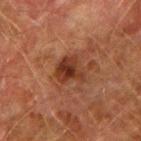<tbp_lesion>
<automated_metrics>
  <cielab_L>30</cielab_L>
  <cielab_a>21</cielab_a>
  <cielab_b>27</cielab_b>
  <vs_skin_darker_L>9.0</vs_skin_darker_L>
  <vs_skin_contrast_norm>9.0</vs_skin_contrast_norm>
  <nevus_likeness_0_100>80</nevus_likeness_0_100>
  <lesion_detection_confidence_0_100>100</lesion_detection_confidence_0_100>
</automated_metrics>
<site>right upper arm</site>
<image>
  <source>total-body photography crop</source>
  <field_of_view_mm>15</field_of_view_mm>
</image>
<patient>
  <sex>male</sex>
  <age_approx>75</age_approx>
</patient>
<lesion_size>
  <long_diameter_mm_approx>3.5</long_diameter_mm_approx>
</lesion_size>
<lighting>cross-polarized</lighting>
</tbp_lesion>